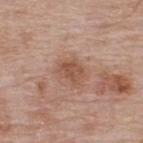Impression:
Recorded during total-body skin imaging; not selected for excision or biopsy.
Clinical summary:
Cropped from a whole-body photographic skin survey; the tile spans about 15 mm. A male subject, in their mid- to late 60s. The lesion is on the upper back. Captured under white-light illumination. Automated image analysis of the tile measured a lesion–skin lightness drop of about 9. The analysis additionally found a border-irregularity rating of about 2.5/10, internal color variation of about 3 on a 0–10 scale, and radial color variation of about 1.